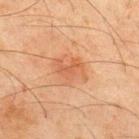Clinical impression:
Imaged during a routine full-body skin examination; the lesion was not biopsied and no histopathology is available.
Acquisition and patient details:
A male subject in their mid-40s. A close-up tile cropped from a whole-body skin photograph, about 15 mm across. On the upper back.